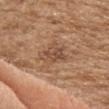A male patient aged 68–72.
Located on the chest.
A 15 mm crop from a total-body photograph taken for skin-cancer surveillance.